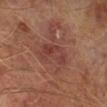follow-up: total-body-photography surveillance lesion; no biopsy
automated metrics: a footprint of about 8 mm², an outline eccentricity of about 0.85 (0 = round, 1 = elongated), and a shape-asymmetry score of about 0.4 (0 = symmetric); a lesion color around L≈39 a*≈24 b*≈24 in CIELAB, roughly 7 lightness units darker than nearby skin, and a normalized border contrast of about 6
anatomic site: the right lower leg
imaging modality: 15 mm crop, total-body photography
illumination: cross-polarized illumination
subject: male, aged approximately 65
size: ~4.5 mm (longest diameter)